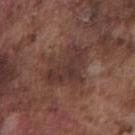The lesion was tiled from a total-body skin photograph and was not biopsied. Located on the chest. Cropped from a total-body skin-imaging series; the visible field is about 15 mm. The subject is a male in their mid- to late 70s.